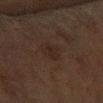Q: Was this lesion biopsied?
A: imaged on a skin check; not biopsied
Q: What are the patient's age and sex?
A: female, in their 50s
Q: How was the tile lit?
A: cross-polarized
Q: Lesion location?
A: the left forearm
Q: Lesion size?
A: ~3 mm (longest diameter)
Q: How was this image acquired?
A: ~15 mm crop, total-body skin-cancer survey
Q: Automated lesion metrics?
A: a shape eccentricity near 0.55